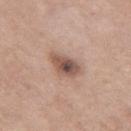follow-up: imaged on a skin check; not biopsied | patient: female, approximately 55 years of age | imaging modality: ~15 mm crop, total-body skin-cancer survey | site: the left thigh.